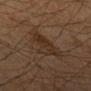<tbp_lesion>
  <biopsy_status>not biopsied; imaged during a skin examination</biopsy_status>
  <automated_metrics>
    <cielab_L>28</cielab_L>
    <cielab_a>15</cielab_a>
    <cielab_b>24</cielab_b>
    <vs_skin_darker_L>6.0</vs_skin_darker_L>
    <vs_skin_contrast_norm>6.5</vs_skin_contrast_norm>
    <border_irregularity_0_10>5.0</border_irregularity_0_10>
    <color_variation_0_10>3.5</color_variation_0_10>
    <peripheral_color_asymmetry>1.0</peripheral_color_asymmetry>
  </automated_metrics>
  <patient>
    <sex>male</sex>
    <age_approx>70</age_approx>
  </patient>
  <lesion_size>
    <long_diameter_mm_approx>4.0</long_diameter_mm_approx>
  </lesion_size>
  <image>
    <source>total-body photography crop</source>
    <field_of_view_mm>15</field_of_view_mm>
  </image>
  <lighting>cross-polarized</lighting>
  <site>left forearm</site>
</tbp_lesion>